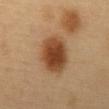Captured during whole-body skin photography for melanoma surveillance; the lesion was not biopsied.
The lesion's longest dimension is about 5.5 mm.
From the abdomen.
A female patient, aged approximately 60.
This is a cross-polarized tile.
A lesion tile, about 15 mm wide, cut from a 3D total-body photograph.